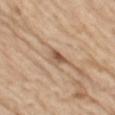workup: total-body-photography surveillance lesion; no biopsy
TBP lesion metrics: an eccentricity of roughly 0.75
lesion diameter: about 3 mm
patient: male, in their 70s
body site: the leg
image: total-body-photography crop, ~15 mm field of view